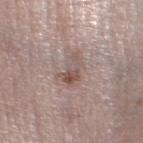This lesion was catalogued during total-body skin photography and was not selected for biopsy. Measured at roughly 4 mm in maximum diameter. From the left lower leg. Captured under white-light illumination. An algorithmic analysis of the crop reported a lesion area of about 8 mm² and two-axis asymmetry of about 0.3. The analysis additionally found a border-irregularity index near 3.5/10, internal color variation of about 9.5 on a 0–10 scale, and peripheral color asymmetry of about 3. A 15 mm close-up tile from a total-body photography series done for melanoma screening. A female patient aged 48 to 52.